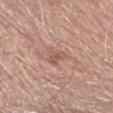biopsy_status: not biopsied; imaged during a skin examination
patient:
  sex: male
  age_approx: 65
lighting: white-light
site: right forearm
lesion_size:
  long_diameter_mm_approx: 3.0
image:
  source: total-body photography crop
  field_of_view_mm: 15
automated_metrics:
  cielab_L: 56
  cielab_a: 22
  cielab_b: 26
  vs_skin_darker_L: 8.0
  vs_skin_contrast_norm: 5.5